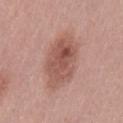The lesion was photographed on a routine skin check and not biopsied; there is no pathology result. A roughly 15 mm field-of-view crop from a total-body skin photograph. Located on the lower back. Imaged with white-light lighting. A female subject, approximately 40 years of age. Longest diameter approximately 6 mm. The total-body-photography lesion software estimated a mean CIELAB color near L≈53 a*≈23 b*≈25, roughly 11 lightness units darker than nearby skin, and a normalized border contrast of about 7.5. The software also gave border irregularity of about 2 on a 0–10 scale, a color-variation rating of about 5.5/10, and a peripheral color-asymmetry measure near 2.